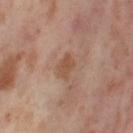Impression:
This lesion was catalogued during total-body skin photography and was not selected for biopsy.
Clinical summary:
A female patient, in their mid- to late 50s. Longest diameter approximately 3 mm. The tile uses cross-polarized illumination. The lesion is located on the leg. Cropped from a total-body skin-imaging series; the visible field is about 15 mm.